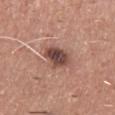No biopsy was performed on this lesion — it was imaged during a full skin examination and was not determined to be concerning.
A roughly 15 mm field-of-view crop from a total-body skin photograph.
The patient is a male approximately 45 years of age.
This is a white-light tile.
The lesion is located on the chest.
Longest diameter approximately 3.5 mm.